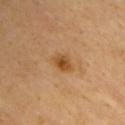Q: Was a biopsy performed?
A: imaged on a skin check; not biopsied
Q: Lesion size?
A: ≈2.5 mm
Q: Illumination type?
A: cross-polarized illumination
Q: How was this image acquired?
A: ~15 mm tile from a whole-body skin photo
Q: What are the patient's age and sex?
A: male, approximately 65 years of age
Q: What did automated image analysis measure?
A: a lesion-detection confidence of about 100/100
Q: Where on the body is the lesion?
A: the chest